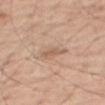No biopsy was performed on this lesion — it was imaged during a full skin examination and was not determined to be concerning. From the leg. Cropped from a total-body skin-imaging series; the visible field is about 15 mm. A male subject aged around 70.